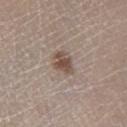Assessment:
This lesion was catalogued during total-body skin photography and was not selected for biopsy.
Context:
A lesion tile, about 15 mm wide, cut from a 3D total-body photograph. The total-body-photography lesion software estimated a border-irregularity rating of about 3.5/10, internal color variation of about 2 on a 0–10 scale, and peripheral color asymmetry of about 0.5. It also reported a detector confidence of about 100 out of 100 that the crop contains a lesion. The lesion's longest dimension is about 3 mm. The lesion is on the leg. Captured under white-light illumination. A female patient, about 45 years old.